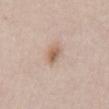{
  "biopsy_status": "not biopsied; imaged during a skin examination",
  "lesion_size": {
    "long_diameter_mm_approx": 2.5
  },
  "lighting": "white-light",
  "site": "abdomen",
  "image": {
    "source": "total-body photography crop",
    "field_of_view_mm": 15
  },
  "patient": {
    "sex": "male",
    "age_approx": 40
  }
}